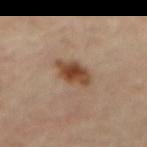{"biopsy_status": "not biopsied; imaged during a skin examination", "patient": {"sex": "female", "age_approx": 65}, "lighting": "cross-polarized", "site": "back", "lesion_size": {"long_diameter_mm_approx": 4.5}, "image": {"source": "total-body photography crop", "field_of_view_mm": 15}}